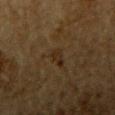  biopsy_status: not biopsied; imaged during a skin examination
  image:
    source: total-body photography crop
    field_of_view_mm: 15
  automated_metrics:
    eccentricity: 0.9
    shape_asymmetry: 0.4
    nevus_likeness_0_100: 35
    lesion_detection_confidence_0_100: 65
  patient:
    sex: male
    age_approx: 85+
  lesion_size:
    long_diameter_mm_approx: 3.0
  lighting: cross-polarized
  site: arm